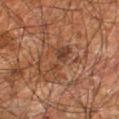Findings:
• notes — catalogued during a skin exam; not biopsied
• lesion size — ~4.5 mm (longest diameter)
• patient — male, aged approximately 60
• anatomic site — the right leg
• image source — total-body-photography crop, ~15 mm field of view